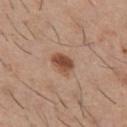Recorded during total-body skin imaging; not selected for excision or biopsy. On the chest. The recorded lesion diameter is about 3 mm. A lesion tile, about 15 mm wide, cut from a 3D total-body photograph. The patient is a male aged around 30. An algorithmic analysis of the crop reported an average lesion color of about L≈49 a*≈21 b*≈30 (CIELAB), roughly 13 lightness units darker than nearby skin, and a normalized lesion–skin contrast near 9.5. The analysis additionally found border irregularity of about 2 on a 0–10 scale and a color-variation rating of about 4/10. It also reported a classifier nevus-likeness of about 95/100.